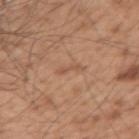- workup — no biopsy performed (imaged during a skin exam)